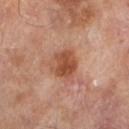Q: Is there a histopathology result?
A: imaged on a skin check; not biopsied
Q: What kind of image is this?
A: total-body-photography crop, ~15 mm field of view
Q: Lesion location?
A: the leg
Q: Who is the patient?
A: male, in their mid- to late 60s